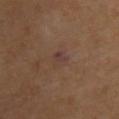Recorded during total-body skin imaging; not selected for excision or biopsy. Located on the upper back. A 15 mm close-up tile from a total-body photography series done for melanoma screening. A male subject, roughly 70 years of age. About 2.5 mm across. The lesion-visualizer software estimated an eccentricity of roughly 0.7. And it measured an average lesion color of about L≈37 a*≈17 b*≈21 (CIELAB), a lesion–skin lightness drop of about 5, and a normalized border contrast of about 6. The software also gave a color-variation rating of about 4/10 and peripheral color asymmetry of about 1.5.